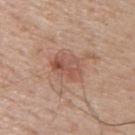Q: Was a biopsy performed?
A: total-body-photography surveillance lesion; no biopsy
Q: Where on the body is the lesion?
A: the upper back
Q: Patient demographics?
A: male, about 70 years old
Q: How was this image acquired?
A: 15 mm crop, total-body photography